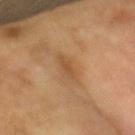<lesion>
<image>
  <source>total-body photography crop</source>
  <field_of_view_mm>15</field_of_view_mm>
</image>
<lesion_size>
  <long_diameter_mm_approx>3.5</long_diameter_mm_approx>
</lesion_size>
<lighting>cross-polarized</lighting>
<patient>
  <sex>male</sex>
  <age_approx>65</age_approx>
</patient>
</lesion>